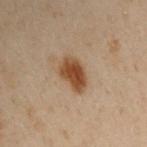This lesion was catalogued during total-body skin photography and was not selected for biopsy.
Captured under cross-polarized illumination.
The recorded lesion diameter is about 4 mm.
A male subject roughly 50 years of age.
A 15 mm close-up extracted from a 3D total-body photography capture.
The lesion is located on the left arm.